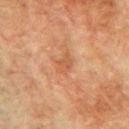| field | value |
|---|---|
| notes | total-body-photography surveillance lesion; no biopsy |
| site | the right upper arm |
| patient | male, roughly 75 years of age |
| image source | ~15 mm tile from a whole-body skin photo |
| tile lighting | cross-polarized |